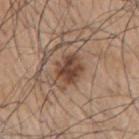acquisition: total-body-photography crop, ~15 mm field of view
tile lighting: white-light illumination
diameter: ~3 mm (longest diameter)
patient: male, aged around 75
body site: the right upper arm